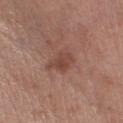- notes — no biopsy performed (imaged during a skin exam)
- lighting — white-light
- body site — the right lower leg
- lesion size — ≈3.5 mm
- acquisition — total-body-photography crop, ~15 mm field of view
- patient — female, aged 38–42
- TBP lesion metrics — an area of roughly 5.5 mm², an eccentricity of roughly 0.75, and a shape-asymmetry score of about 0.3 (0 = symmetric); a classifier nevus-likeness of about 10/100 and a detector confidence of about 100 out of 100 that the crop contains a lesion